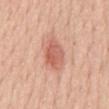Clinical summary:
Cropped from a total-body skin-imaging series; the visible field is about 15 mm. Longest diameter approximately 4 mm. From the back. This is a white-light tile. The patient is a female in their mid- to late 50s.